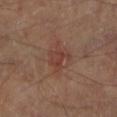– workup — catalogued during a skin exam; not biopsied
– image-analysis metrics — a footprint of about 6.5 mm² and two-axis asymmetry of about 0.4; an average lesion color of about L≈39 a*≈21 b*≈24 (CIELAB), about 6 CIELAB-L* units darker than the surrounding skin, and a normalized border contrast of about 5.5; a border-irregularity rating of about 5/10, internal color variation of about 3.5 on a 0–10 scale, and a peripheral color-asymmetry measure near 1.5
– patient — male, aged approximately 70
– anatomic site — the left lower leg
– lesion diameter — ≈3 mm
– acquisition — total-body-photography crop, ~15 mm field of view
– lighting — cross-polarized illumination